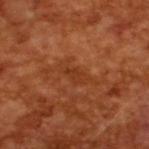{
  "biopsy_status": "not biopsied; imaged during a skin examination",
  "automated_metrics": {
    "cielab_L": 36,
    "cielab_a": 27,
    "cielab_b": 36,
    "vs_skin_darker_L": 6.0,
    "vs_skin_contrast_norm": 5.0
  },
  "patient": {
    "sex": "male",
    "age_approx": 65
  },
  "image": {
    "source": "total-body photography crop",
    "field_of_view_mm": 15
  },
  "lighting": "cross-polarized"
}